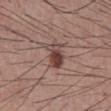Findings:
– notes — catalogued during a skin exam; not biopsied
– tile lighting — white-light
– patient — male, roughly 40 years of age
– image source — ~15 mm tile from a whole-body skin photo
– anatomic site — the abdomen
– TBP lesion metrics — an area of roughly 7 mm² and two-axis asymmetry of about 0.25; an average lesion color of about L≈43 a*≈18 b*≈22 (CIELAB) and a lesion–skin lightness drop of about 12; a border-irregularity index near 2.5/10 and internal color variation of about 7.5 on a 0–10 scale; a nevus-likeness score of about 95/100 and a detector confidence of about 100 out of 100 that the crop contains a lesion